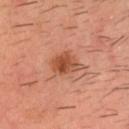| feature | finding |
|---|---|
| notes | catalogued during a skin exam; not biopsied |
| location | the head or neck |
| TBP lesion metrics | a nevus-likeness score of about 95/100 and a lesion-detection confidence of about 100/100 |
| lesion diameter | about 3.5 mm |
| image | total-body-photography crop, ~15 mm field of view |
| tile lighting | cross-polarized |
| subject | male, aged 33 to 37 |